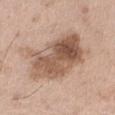Part of a total-body skin-imaging series; this lesion was reviewed on a skin check and was not flagged for biopsy.
Located on the right thigh.
A male patient, approximately 50 years of age.
Imaged with white-light lighting.
A 15 mm crop from a total-body photograph taken for skin-cancer surveillance.
The recorded lesion diameter is about 7 mm.
The lesion-visualizer software estimated border irregularity of about 3 on a 0–10 scale and a within-lesion color-variation index near 8.5/10. And it measured an automated nevus-likeness rating near 10 out of 100 and a detector confidence of about 100 out of 100 that the crop contains a lesion.